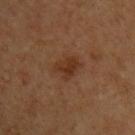The lesion was tiled from a total-body skin photograph and was not biopsied. Captured under cross-polarized illumination. Measured at roughly 3 mm in maximum diameter. From the arm. The patient is a male in their 60s. A 15 mm close-up extracted from a 3D total-body photography capture.